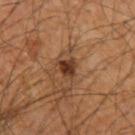notes: no biopsy performed (imaged during a skin exam) | body site: the arm | subject: male, in their mid-60s | lesion diameter: ≈2.5 mm | automated metrics: a lesion area of about 4 mm², an outline eccentricity of about 0.45 (0 = round, 1 = elongated), and two-axis asymmetry of about 0.4; a mean CIELAB color near L≈35 a*≈20 b*≈29, a lesion–skin lightness drop of about 13, and a normalized border contrast of about 11; border irregularity of about 4 on a 0–10 scale, a within-lesion color-variation index near 2.5/10, and peripheral color asymmetry of about 1; a lesion-detection confidence of about 100/100 | lighting: cross-polarized illumination | image: total-body-photography crop, ~15 mm field of view.